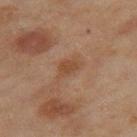Assessment:
Captured during whole-body skin photography for melanoma surveillance; the lesion was not biopsied.
Background:
The subject is a female aged 58 to 62. The total-body-photography lesion software estimated an average lesion color of about L≈42 a*≈18 b*≈30 (CIELAB). It also reported a border-irregularity index near 3/10. A roughly 15 mm field-of-view crop from a total-body skin photograph. Imaged with cross-polarized lighting. The lesion is on the right thigh. Longest diameter approximately 3 mm.